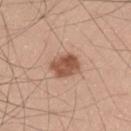Q: Is there a histopathology result?
A: total-body-photography surveillance lesion; no biopsy
Q: Who is the patient?
A: male, about 30 years old
Q: What is the imaging modality?
A: total-body-photography crop, ~15 mm field of view
Q: What is the anatomic site?
A: the left thigh